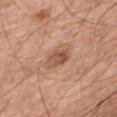  biopsy_status: not biopsied; imaged during a skin examination
  lesion_size:
    long_diameter_mm_approx: 3.0
  image:
    source: total-body photography crop
    field_of_view_mm: 15
  patient:
    sex: male
    age_approx: 80
  site: upper back
  lighting: white-light
  automated_metrics:
    area_mm2_approx: 5.5
    eccentricity: 0.75
    shape_asymmetry: 0.25
    cielab_L: 53
    cielab_a: 23
    cielab_b: 32
    nevus_likeness_0_100: 10
    lesion_detection_confidence_0_100: 100